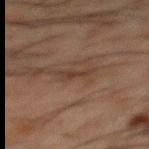Case summary:
- workup: no biopsy performed (imaged during a skin exam)
- acquisition: total-body-photography crop, ~15 mm field of view
- patient: male, in their 50s
- lesion diameter: ~3 mm (longest diameter)
- site: the mid back
- lighting: cross-polarized illumination
- automated lesion analysis: an eccentricity of roughly 0.95 and two-axis asymmetry of about 0.45; an average lesion color of about L≈28 a*≈14 b*≈20 (CIELAB) and about 6 CIELAB-L* units darker than the surrounding skin; a border-irregularity index near 5/10, a within-lesion color-variation index near 0/10, and peripheral color asymmetry of about 0